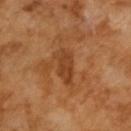Case summary:
- follow-up · imaged on a skin check; not biopsied
- lighting · cross-polarized illumination
- subject · male, roughly 65 years of age
- acquisition · ~15 mm crop, total-body skin-cancer survey
- lesion size · about 5 mm